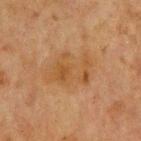<lesion>
  <biopsy_status>not biopsied; imaged during a skin examination</biopsy_status>
  <lighting>cross-polarized</lighting>
  <automated_metrics>
    <cielab_L>42</cielab_L>
    <cielab_a>17</cielab_a>
    <cielab_b>32</cielab_b>
    <border_irregularity_0_10>3.0</border_irregularity_0_10>
    <color_variation_0_10>4.0</color_variation_0_10>
    <peripheral_color_asymmetry>1.5</peripheral_color_asymmetry>
  </automated_metrics>
  <lesion_size>
    <long_diameter_mm_approx>6.0</long_diameter_mm_approx>
  </lesion_size>
  <site>chest</site>
  <image>
    <source>total-body photography crop</source>
    <field_of_view_mm>15</field_of_view_mm>
  </image>
  <patient>
    <sex>male</sex>
    <age_approx>65</age_approx>
  </patient>
</lesion>